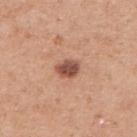Imaged during a routine full-body skin examination; the lesion was not biopsied and no histopathology is available.
The lesion is on the arm.
The patient is a female aged approximately 40.
The tile uses white-light illumination.
About 2.5 mm across.
A close-up tile cropped from a whole-body skin photograph, about 15 mm across.
Automated image analysis of the tile measured a lesion color around L≈51 a*≈24 b*≈29 in CIELAB, a lesion–skin lightness drop of about 15, and a normalized border contrast of about 10.